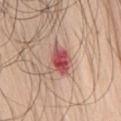notes — catalogued during a skin exam; not biopsied | image — ~15 mm tile from a whole-body skin photo | diameter — about 5 mm | body site — the chest | illumination — white-light | subject — male, roughly 70 years of age | automated lesion analysis — internal color variation of about 8.5 on a 0–10 scale and peripheral color asymmetry of about 3.